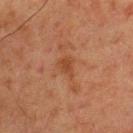notes: imaged on a skin check; not biopsied | acquisition: ~15 mm tile from a whole-body skin photo | body site: the upper back | subject: male, approximately 60 years of age.